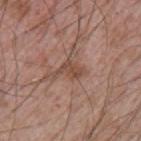Background: A close-up tile cropped from a whole-body skin photograph, about 15 mm across. A male subject, about 65 years old. Captured under white-light illumination. The recorded lesion diameter is about 3.5 mm. The lesion is on the left upper arm.